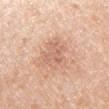Clinical impression:
Recorded during total-body skin imaging; not selected for excision or biopsy.
Image and clinical context:
From the right upper arm. About 3 mm across. The patient is a female in their 50s. A close-up tile cropped from a whole-body skin photograph, about 15 mm across. Imaged with white-light lighting. Automated tile analysis of the lesion measured an average lesion color of about L≈64 a*≈23 b*≈30 (CIELAB) and a lesion–skin lightness drop of about 8.